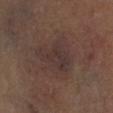The lesion was tiled from a total-body skin photograph and was not biopsied.
Cropped from a whole-body photographic skin survey; the tile spans about 15 mm.
Automated image analysis of the tile measured a footprint of about 11 mm², an eccentricity of roughly 0.75, and two-axis asymmetry of about 0.4. It also reported an average lesion color of about L≈33 a*≈15 b*≈18 (CIELAB), about 5 CIELAB-L* units darker than the surrounding skin, and a lesion-to-skin contrast of about 6 (normalized; higher = more distinct).
The subject is female.
From the left lower leg.
Imaged with cross-polarized lighting.
Longest diameter approximately 5 mm.